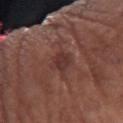follow-up: catalogued during a skin exam; not biopsied
anatomic site: the right forearm
patient: female, in their mid-70s
lighting: white-light illumination
image: ~15 mm tile from a whole-body skin photo